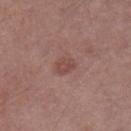follow-up: total-body-photography surveillance lesion; no biopsy
lesion size: about 2.5 mm
patient: male, about 70 years old
image: ~15 mm tile from a whole-body skin photo
lighting: white-light
automated metrics: a lesion area of about 4.5 mm², a shape eccentricity near 0.65, and a symmetry-axis asymmetry near 0.15; a nevus-likeness score of about 15/100 and a lesion-detection confidence of about 100/100
site: the leg